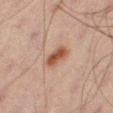biopsy status = total-body-photography surveillance lesion; no biopsy
lesion diameter = ~3.5 mm (longest diameter)
subject = male, aged 58 to 62
image = ~15 mm crop, total-body skin-cancer survey
anatomic site = the left leg